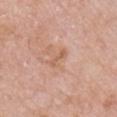Clinical impression: The lesion was tiled from a total-body skin photograph and was not biopsied. Clinical summary: A close-up tile cropped from a whole-body skin photograph, about 15 mm across. A male subject approximately 60 years of age. Longest diameter approximately 3 mm. From the right upper arm.